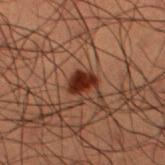{"biopsy_status": "not biopsied; imaged during a skin examination", "site": "left lower leg", "automated_metrics": {"area_mm2_approx": 5.5, "shape_asymmetry": 0.3, "cielab_L": 23, "cielab_a": 21, "cielab_b": 24, "vs_skin_darker_L": 13.0, "vs_skin_contrast_norm": 13.0, "color_variation_0_10": 2.5, "nevus_likeness_0_100": 95, "lesion_detection_confidence_0_100": 100}, "patient": {"sex": "male", "age_approx": 50}, "image": {"source": "total-body photography crop", "field_of_view_mm": 15}}